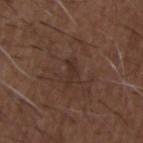Impression:
Imaged during a routine full-body skin examination; the lesion was not biopsied and no histopathology is available.